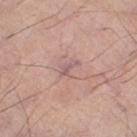The lesion was photographed on a routine skin check and not biopsied; there is no pathology result. Located on the left thigh. A male patient, aged 68–72. A 15 mm close-up extracted from a 3D total-body photography capture.